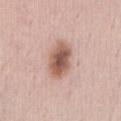{"patient": {"sex": "female", "age_approx": 40}, "lighting": "white-light", "image": {"source": "total-body photography crop", "field_of_view_mm": 15}, "site": "front of the torso"}